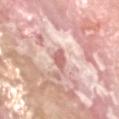No biopsy was performed on this lesion — it was imaged during a full skin examination and was not determined to be concerning. A 15 mm close-up extracted from a 3D total-body photography capture. The lesion-visualizer software estimated an area of roughly 3.5 mm² and an eccentricity of roughly 0.75. A male subject aged around 65. About 2.5 mm across. Captured under white-light illumination. Located on the head or neck.